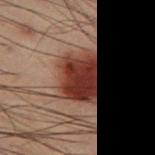follow-up: imaged on a skin check; not biopsied | image source: total-body-photography crop, ~15 mm field of view | location: the left thigh | tile lighting: cross-polarized | automated lesion analysis: a lesion area of about 20 mm² and an outline eccentricity of about 0.65 (0 = round, 1 = elongated); a border-irregularity rating of about 2.5/10 and peripheral color asymmetry of about 2.5; an automated nevus-likeness rating near 100 out of 100 and lesion-presence confidence of about 80/100 | patient: male, aged 53 to 57.